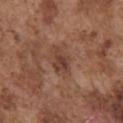notes=total-body-photography surveillance lesion; no biopsy | location=the front of the torso | patient=male, in their mid- to late 70s | imaging modality=~15 mm crop, total-body skin-cancer survey | tile lighting=white-light | lesion diameter=≈3 mm.